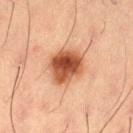The lesion was tiled from a total-body skin photograph and was not biopsied. A roughly 15 mm field-of-view crop from a total-body skin photograph. The subject is a male aged around 55. From the right thigh. Approximately 4.5 mm at its widest. The lesion-visualizer software estimated a footprint of about 13 mm² and two-axis asymmetry of about 0.15. It also reported an average lesion color of about L≈46 a*≈24 b*≈33 (CIELAB), a lesion–skin lightness drop of about 16, and a normalized lesion–skin contrast near 12. It also reported a border-irregularity index near 1.5/10, internal color variation of about 6.5 on a 0–10 scale, and a peripheral color-asymmetry measure near 2. The software also gave an automated nevus-likeness rating near 100 out of 100 and a detector confidence of about 100 out of 100 that the crop contains a lesion. This is a cross-polarized tile.